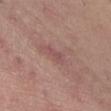Recorded during total-body skin imaging; not selected for excision or biopsy.